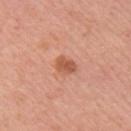Imaged during a routine full-body skin examination; the lesion was not biopsied and no histopathology is available. On the right upper arm. A close-up tile cropped from a whole-body skin photograph, about 15 mm across. Approximately 2.5 mm at its widest. A female patient aged approximately 55. An algorithmic analysis of the crop reported a mean CIELAB color near L≈56 a*≈27 b*≈34.